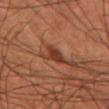Captured during whole-body skin photography for melanoma surveillance; the lesion was not biopsied. Imaged with cross-polarized lighting. Longest diameter approximately 2.5 mm. A male subject, aged 58–62. The lesion is located on the left forearm. This image is a 15 mm lesion crop taken from a total-body photograph.